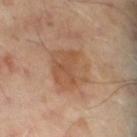workup: total-body-photography surveillance lesion; no biopsy
illumination: cross-polarized illumination
subject: male, roughly 50 years of age
location: the abdomen
size: about 5 mm
TBP lesion metrics: an area of roughly 16 mm² and a symmetry-axis asymmetry near 0.15; an average lesion color of about L≈52 a*≈20 b*≈32 (CIELAB), a lesion–skin lightness drop of about 8, and a lesion-to-skin contrast of about 6 (normalized; higher = more distinct); a border-irregularity rating of about 2/10, a within-lesion color-variation index near 3.5/10, and a peripheral color-asymmetry measure near 1; a nevus-likeness score of about 45/100 and a lesion-detection confidence of about 100/100
image: ~15 mm tile from a whole-body skin photo